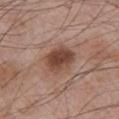Clinical impression: This lesion was catalogued during total-body skin photography and was not selected for biopsy. Context: A 15 mm crop from a total-body photograph taken for skin-cancer surveillance. Measured at roughly 4 mm in maximum diameter. Captured under white-light illumination. The subject is a male aged around 65. The lesion is on the chest.